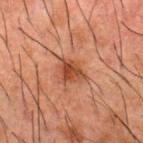Case summary:
* workup — catalogued during a skin exam; not biopsied
* body site — the upper back
* subject — male, approximately 50 years of age
* tile lighting — cross-polarized illumination
* lesion diameter — about 3 mm
* acquisition — ~15 mm tile from a whole-body skin photo